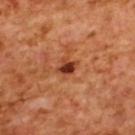Part of a total-body skin-imaging series; this lesion was reviewed on a skin check and was not flagged for biopsy. The lesion is located on the upper back. The tile uses cross-polarized illumination. The total-body-photography lesion software estimated a lesion area of about 3.5 mm² and two-axis asymmetry of about 0.25. The analysis additionally found a mean CIELAB color near L≈40 a*≈31 b*≈37, about 15 CIELAB-L* units darker than the surrounding skin, and a normalized border contrast of about 11.5. The analysis additionally found border irregularity of about 2.5 on a 0–10 scale and peripheral color asymmetry of about 0.5. Cropped from a whole-body photographic skin survey; the tile spans about 15 mm. The recorded lesion diameter is about 2.5 mm. A female patient, aged approximately 55.